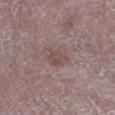Impression: This lesion was catalogued during total-body skin photography and was not selected for biopsy. Background: Automated image analysis of the tile measured a lesion color around L≈47 a*≈17 b*≈18 in CIELAB and roughly 7 lightness units darker than nearby skin. It also reported a nevus-likeness score of about 5/100 and lesion-presence confidence of about 100/100. On the left lower leg. A 15 mm crop from a total-body photograph taken for skin-cancer surveillance. Imaged with white-light lighting. Measured at roughly 2.5 mm in maximum diameter. A female subject, roughly 65 years of age.